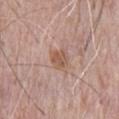{"biopsy_status": "not biopsied; imaged during a skin examination", "site": "chest", "lesion_size": {"long_diameter_mm_approx": 2.5}, "patient": {"sex": "male", "age_approx": 70}, "image": {"source": "total-body photography crop", "field_of_view_mm": 15}}